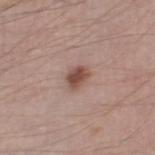Impression:
No biopsy was performed on this lesion — it was imaged during a full skin examination and was not determined to be concerning.
Background:
A close-up tile cropped from a whole-body skin photograph, about 15 mm across. A male subject roughly 40 years of age. The lesion is located on the right thigh. Approximately 2.5 mm at its widest.